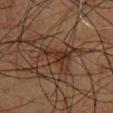Recorded during total-body skin imaging; not selected for excision or biopsy. Cropped from a total-body skin-imaging series; the visible field is about 15 mm. A male subject in their 60s. The recorded lesion diameter is about 4 mm. An algorithmic analysis of the crop reported an area of roughly 5 mm², an eccentricity of roughly 0.9, and two-axis asymmetry of about 0.45. The analysis additionally found a mean CIELAB color near L≈23 a*≈15 b*≈22, about 6 CIELAB-L* units darker than the surrounding skin, and a lesion-to-skin contrast of about 7 (normalized; higher = more distinct). And it measured border irregularity of about 5 on a 0–10 scale, a color-variation rating of about 4/10, and peripheral color asymmetry of about 1.5. This is a cross-polarized tile. Located on the chest.